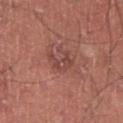anatomic site: the right lower leg
lesion size: about 3.5 mm
tile lighting: white-light
imaging modality: 15 mm crop, total-body photography
patient: male, approximately 45 years of age
TBP lesion metrics: an area of roughly 5 mm² and a symmetry-axis asymmetry near 0.55; an average lesion color of about L≈45 a*≈25 b*≈24 (CIELAB), a lesion–skin lightness drop of about 7, and a lesion-to-skin contrast of about 6 (normalized; higher = more distinct); border irregularity of about 6.5 on a 0–10 scale, a within-lesion color-variation index near 1.5/10, and peripheral color asymmetry of about 0.5